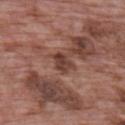follow-up — total-body-photography surveillance lesion; no biopsy
subject — male, aged 68 to 72
illumination — white-light
image-analysis metrics — a border-irregularity index near 2.5/10 and a peripheral color-asymmetry measure near 1.5
site — the back
acquisition — ~15 mm tile from a whole-body skin photo